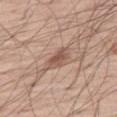Part of a total-body skin-imaging series; this lesion was reviewed on a skin check and was not flagged for biopsy. A male patient aged 53–57. A roughly 15 mm field-of-view crop from a total-body skin photograph. Measured at roughly 3.5 mm in maximum diameter. Located on the leg. The tile uses white-light illumination.